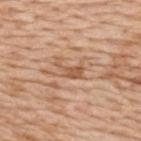Impression: No biopsy was performed on this lesion — it was imaged during a full skin examination and was not determined to be concerning. Background: The lesion is located on the upper back. An algorithmic analysis of the crop reported internal color variation of about 1 on a 0–10 scale and a peripheral color-asymmetry measure near 0. It also reported an automated nevus-likeness rating near 0 out of 100 and lesion-presence confidence of about 65/100. A female patient aged around 45. The recorded lesion diameter is about 3.5 mm. A region of skin cropped from a whole-body photographic capture, roughly 15 mm wide. Captured under white-light illumination.